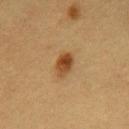Case summary:
- follow-up · no biopsy performed (imaged during a skin exam)
- tile lighting · cross-polarized
- patient · female, roughly 55 years of age
- diameter · ~3 mm (longest diameter)
- image · 15 mm crop, total-body photography
- body site · the chest
- automated metrics · an outline eccentricity of about 0.8 (0 = round, 1 = elongated); an automated nevus-likeness rating near 100 out of 100 and lesion-presence confidence of about 100/100